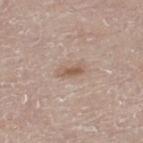biopsy_status: not biopsied; imaged during a skin examination
automated_metrics:
  area_mm2_approx: 3.5
  eccentricity: 0.9
  nevus_likeness_0_100: 60
  lesion_detection_confidence_0_100: 100
lesion_size:
  long_diameter_mm_approx: 3.0
patient:
  sex: male
  age_approx: 80
site: left thigh
image:
  source: total-body photography crop
  field_of_view_mm: 15